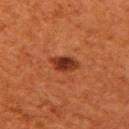Case summary:
– workup · no biopsy performed (imaged during a skin exam)
– lighting · cross-polarized illumination
– site · the right upper arm
– image-analysis metrics · an area of roughly 5.5 mm², a shape eccentricity near 0.8, and two-axis asymmetry of about 0.25; an average lesion color of about L≈37 a*≈31 b*≈36 (CIELAB), about 13 CIELAB-L* units darker than the surrounding skin, and a lesion-to-skin contrast of about 10.5 (normalized; higher = more distinct)
– lesion diameter · ≈3.5 mm
– acquisition · total-body-photography crop, ~15 mm field of view
– patient · female, aged approximately 65